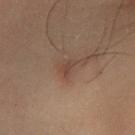Clinical impression:
Captured during whole-body skin photography for melanoma surveillance; the lesion was not biopsied.
Context:
Automated tile analysis of the lesion measured a lesion area of about 3 mm², an outline eccentricity of about 0.75 (0 = round, 1 = elongated), and a symmetry-axis asymmetry near 0.4. The analysis additionally found a lesion color around L≈34 a*≈14 b*≈21 in CIELAB, about 5 CIELAB-L* units darker than the surrounding skin, and a lesion-to-skin contrast of about 5.5 (normalized; higher = more distinct). A lesion tile, about 15 mm wide, cut from a 3D total-body photograph. Located on the left lower leg. The subject is a male aged 53–57. Captured under cross-polarized illumination.